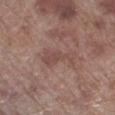Q: Was a biopsy performed?
A: total-body-photography surveillance lesion; no biopsy
Q: What lighting was used for the tile?
A: white-light illumination
Q: What is the lesion's diameter?
A: about 4.5 mm
Q: What kind of image is this?
A: ~15 mm tile from a whole-body skin photo
Q: What is the anatomic site?
A: the leg
Q: Who is the patient?
A: male, about 70 years old